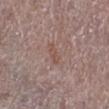Assessment:
No biopsy was performed on this lesion — it was imaged during a full skin examination and was not determined to be concerning.
Background:
Automated image analysis of the tile measured an area of roughly 3.5 mm², an eccentricity of roughly 0.9, and two-axis asymmetry of about 0.25. The analysis additionally found a nevus-likeness score of about 0/100. A female subject aged around 65. The lesion is on the right lower leg. The recorded lesion diameter is about 3 mm. Cropped from a total-body skin-imaging series; the visible field is about 15 mm.